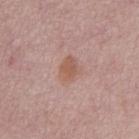<record>
  <biopsy_status>not biopsied; imaged during a skin examination</biopsy_status>
  <lighting>white-light</lighting>
  <site>abdomen</site>
  <patient>
    <sex>male</sex>
    <age_approx>75</age_approx>
  </patient>
  <image>
    <source>total-body photography crop</source>
    <field_of_view_mm>15</field_of_view_mm>
  </image>
</record>